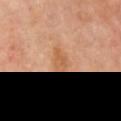patient:
  sex: male
  age_approx: 70
lighting: cross-polarized
image:
  source: total-body photography crop
  field_of_view_mm: 15
lesion_size:
  long_diameter_mm_approx: 3.0
site: back